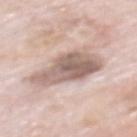Clinical impression:
Captured during whole-body skin photography for melanoma surveillance; the lesion was not biopsied.
Clinical summary:
Cropped from a whole-body photographic skin survey; the tile spans about 15 mm. The subject is a male in their 80s. The total-body-photography lesion software estimated a footprint of about 20 mm², an eccentricity of roughly 0.8, and a symmetry-axis asymmetry near 0.3. On the mid back.